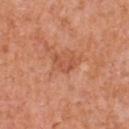Imaged during a routine full-body skin examination; the lesion was not biopsied and no histopathology is available.
A 15 mm close-up tile from a total-body photography series done for melanoma screening.
Imaged with white-light lighting.
A male subject, aged 53 to 57.
About 2.5 mm across.
On the chest.